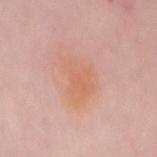notes: imaged on a skin check; not biopsied | patient: female, aged 83 or over | acquisition: ~15 mm crop, total-body skin-cancer survey | location: the front of the torso | automated lesion analysis: a lesion area of about 13 mm², a shape eccentricity near 0.7, and a symmetry-axis asymmetry near 0.4; internal color variation of about 3.5 on a 0–10 scale and radial color variation of about 1; a detector confidence of about 100 out of 100 that the crop contains a lesion.